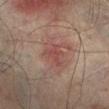Q: Is there a histopathology result?
A: no biopsy performed (imaged during a skin exam)
Q: Lesion location?
A: the right lower leg
Q: What did automated image analysis measure?
A: a lesion area of about 4 mm², an eccentricity of roughly 0.8, and two-axis asymmetry of about 0.55
Q: What are the patient's age and sex?
A: male, approximately 75 years of age
Q: How was this image acquired?
A: ~15 mm crop, total-body skin-cancer survey